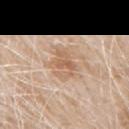Captured during whole-body skin photography for melanoma surveillance; the lesion was not biopsied. The lesion is on the left upper arm. Cropped from a whole-body photographic skin survey; the tile spans about 15 mm. A male patient aged around 80.